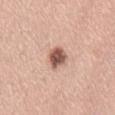Imaged during a routine full-body skin examination; the lesion was not biopsied and no histopathology is available.
This image is a 15 mm lesion crop taken from a total-body photograph.
A female subject, in their 30s.
The total-body-photography lesion software estimated an area of roughly 6 mm² and two-axis asymmetry of about 0.25. It also reported a mean CIELAB color near L≈56 a*≈21 b*≈26 and a normalized border contrast of about 10.5. It also reported a nevus-likeness score of about 90/100 and a lesion-detection confidence of about 100/100.
Located on the lower back.